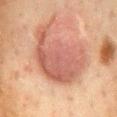<lesion>
<biopsy_status>not biopsied; imaged during a skin examination</biopsy_status>
<site>mid back</site>
<automated_metrics>
  <cielab_L>48</cielab_L>
  <cielab_a>22</cielab_a>
  <cielab_b>25</cielab_b>
  <vs_skin_darker_L>9.0</vs_skin_darker_L>
  <vs_skin_contrast_norm>7.0</vs_skin_contrast_norm>
  <lesion_detection_confidence_0_100>50</lesion_detection_confidence_0_100>
</automated_metrics>
<patient>
  <sex>female</sex>
  <age_approx>50</age_approx>
</patient>
<image>
  <source>total-body photography crop</source>
  <field_of_view_mm>15</field_of_view_mm>
</image>
</lesion>